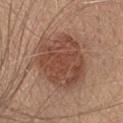Recorded during total-body skin imaging; not selected for excision or biopsy.
A roughly 15 mm field-of-view crop from a total-body skin photograph.
The subject is a female aged 33 to 37.
The lesion is on the head or neck.
Automated tile analysis of the lesion measured an area of roughly 36 mm², an eccentricity of roughly 0.35, and two-axis asymmetry of about 0.25. The analysis additionally found roughly 11 lightness units darker than nearby skin and a normalized border contrast of about 8. The software also gave a nevus-likeness score of about 85/100 and lesion-presence confidence of about 100/100.
Imaged with white-light lighting.
Approximately 7 mm at its widest.